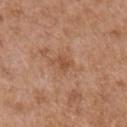Notes:
• workup · total-body-photography surveillance lesion; no biopsy
• acquisition · 15 mm crop, total-body photography
• TBP lesion metrics · a footprint of about 3.5 mm², an outline eccentricity of about 0.75 (0 = round, 1 = elongated), and a symmetry-axis asymmetry near 0.45; border irregularity of about 4.5 on a 0–10 scale, a color-variation rating of about 1/10, and radial color variation of about 0.5; a nevus-likeness score of about 0/100 and lesion-presence confidence of about 100/100
• subject · male, aged approximately 75
• illumination · white-light illumination
• location · the right upper arm